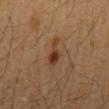biopsy status: no biopsy performed (imaged during a skin exam)
acquisition: total-body-photography crop, ~15 mm field of view
diameter: ≈4 mm
subject: male, aged 33–37
site: the mid back
illumination: cross-polarized